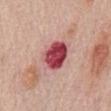<record>
  <biopsy_status>not biopsied; imaged during a skin examination</biopsy_status>
  <image>
    <source>total-body photography crop</source>
    <field_of_view_mm>15</field_of_view_mm>
  </image>
  <patient>
    <sex>female</sex>
    <age_approx>65</age_approx>
  </patient>
  <lighting>white-light</lighting>
  <site>front of the torso</site>
  <automated_metrics>
    <area_mm2_approx>12.0</area_mm2_approx>
    <eccentricity>0.65</eccentricity>
    <shape_asymmetry>0.2</shape_asymmetry>
    <cielab_L>49</cielab_L>
    <cielab_a>35</cielab_a>
    <cielab_b>22</cielab_b>
    <vs_skin_darker_L>19.0</vs_skin_darker_L>
    <vs_skin_contrast_norm>13.0</vs_skin_contrast_norm>
    <color_variation_0_10>7.5</color_variation_0_10>
    <peripheral_color_asymmetry>2.5</peripheral_color_asymmetry>
    <nevus_likeness_0_100>0</nevus_likeness_0_100>
  </automated_metrics>
</record>